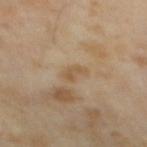biopsy status = catalogued during a skin exam; not biopsied | size = ~2.5 mm (longest diameter) | imaging modality = total-body-photography crop, ~15 mm field of view | patient = male, aged around 65 | illumination = cross-polarized illumination.